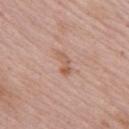Part of a total-body skin-imaging series; this lesion was reviewed on a skin check and was not flagged for biopsy. The lesion's longest dimension is about 3 mm. A roughly 15 mm field-of-view crop from a total-body skin photograph. The patient is a male in their mid-60s. Captured under white-light illumination. The lesion is located on the arm.